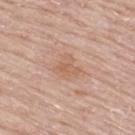Imaged during a routine full-body skin examination; the lesion was not biopsied and no histopathology is available.
The lesion is on the upper back.
The lesion-visualizer software estimated an area of roughly 4.5 mm² and an outline eccentricity of about 0.5 (0 = round, 1 = elongated). It also reported a lesion–skin lightness drop of about 7 and a normalized lesion–skin contrast near 5.5. The software also gave a classifier nevus-likeness of about 0/100 and a lesion-detection confidence of about 100/100.
A male patient approximately 80 years of age.
This is a white-light tile.
A lesion tile, about 15 mm wide, cut from a 3D total-body photograph.
About 3 mm across.